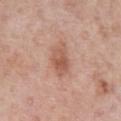  lighting: white-light
  site: chest
  lesion_size:
    long_diameter_mm_approx: 4.0
  patient:
    sex: male
    age_approx: 60
  image:
    source: total-body photography crop
    field_of_view_mm: 15
  automated_metrics:
    cielab_L: 56
    cielab_a: 23
    cielab_b: 30
    border_irregularity_0_10: 2.5
    color_variation_0_10: 4.5
    peripheral_color_asymmetry: 1.5